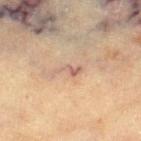| feature | finding |
|---|---|
| follow-up | no biopsy performed (imaged during a skin exam) |
| subject | female, aged 78 to 82 |
| image | 15 mm crop, total-body photography |
| anatomic site | the right thigh |